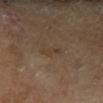Captured during whole-body skin photography for melanoma surveillance; the lesion was not biopsied. A close-up tile cropped from a whole-body skin photograph, about 15 mm across. A male subject, aged approximately 65. The lesion is on the right lower leg.